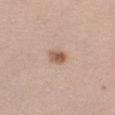Q: Was a biopsy performed?
A: catalogued during a skin exam; not biopsied
Q: What did automated image analysis measure?
A: an area of roughly 4 mm² and two-axis asymmetry of about 0.25; a lesion color around L≈58 a*≈19 b*≈29 in CIELAB, about 12 CIELAB-L* units darker than the surrounding skin, and a normalized border contrast of about 8; a border-irregularity rating of about 2/10, a color-variation rating of about 5/10, and peripheral color asymmetry of about 1.5; a classifier nevus-likeness of about 95/100
Q: How was this image acquired?
A: 15 mm crop, total-body photography
Q: Patient demographics?
A: male, aged 33 to 37
Q: How large is the lesion?
A: ~2.5 mm (longest diameter)
Q: What is the anatomic site?
A: the right upper arm
Q: What lighting was used for the tile?
A: white-light